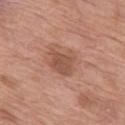A female patient approximately 70 years of age. Cropped from a whole-body photographic skin survey; the tile spans about 15 mm. This is a white-light tile. The lesion's longest dimension is about 3.5 mm. From the leg. The total-body-photography lesion software estimated an area of roughly 8.5 mm², an outline eccentricity of about 0.5 (0 = round, 1 = elongated), and two-axis asymmetry of about 0.3. And it measured an average lesion color of about L≈52 a*≈22 b*≈29 (CIELAB), about 9 CIELAB-L* units darker than the surrounding skin, and a normalized border contrast of about 6.5. The software also gave border irregularity of about 3.5 on a 0–10 scale and peripheral color asymmetry of about 1. And it measured an automated nevus-likeness rating near 20 out of 100 and a detector confidence of about 100 out of 100 that the crop contains a lesion.